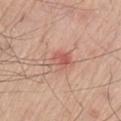Captured during whole-body skin photography for melanoma surveillance; the lesion was not biopsied. Captured under white-light illumination. A male patient, roughly 80 years of age. Approximately 4 mm at its widest. Located on the left thigh. Automated image analysis of the tile measured an outline eccentricity of about 0.85 (0 = round, 1 = elongated) and a symmetry-axis asymmetry near 0.4. The software also gave a classifier nevus-likeness of about 10/100 and a lesion-detection confidence of about 100/100. A roughly 15 mm field-of-view crop from a total-body skin photograph.